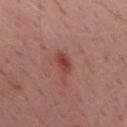<case>
  <biopsy_status>not biopsied; imaged during a skin examination</biopsy_status>
  <image>
    <source>total-body photography crop</source>
    <field_of_view_mm>15</field_of_view_mm>
  </image>
  <lighting>white-light</lighting>
  <lesion_size>
    <long_diameter_mm_approx>3.0</long_diameter_mm_approx>
  </lesion_size>
  <site>mid back</site>
  <patient>
    <sex>male</sex>
    <age_approx>55</age_approx>
  </patient>
</case>